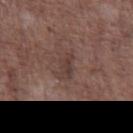• biopsy status · catalogued during a skin exam; not biopsied
• subject · male, aged approximately 70
• size · about 3 mm
• body site · the abdomen
• imaging modality · total-body-photography crop, ~15 mm field of view
• tile lighting · white-light illumination